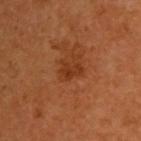  biopsy_status: not biopsied; imaged during a skin examination
  image:
    source: total-body photography crop
    field_of_view_mm: 15
  patient:
    sex: male
    age_approx: 60
  automated_metrics:
    eccentricity: 0.65
    vs_skin_darker_L: 7.0
    vs_skin_contrast_norm: 6.5
  lighting: cross-polarized
  site: upper back